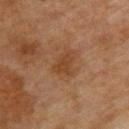Recorded during total-body skin imaging; not selected for excision or biopsy. A 15 mm close-up extracted from a 3D total-body photography capture. On the back. Captured under cross-polarized illumination. A female patient, roughly 70 years of age.